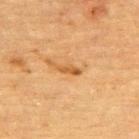Part of a total-body skin-imaging series; this lesion was reviewed on a skin check and was not flagged for biopsy. On the upper back. A female patient aged 68–72. The recorded lesion diameter is about 3 mm. This image is a 15 mm lesion crop taken from a total-body photograph. Automated tile analysis of the lesion measured a lesion area of about 3 mm², an eccentricity of roughly 0.9, and a shape-asymmetry score of about 0.35 (0 = symmetric). The software also gave a lesion color around L≈49 a*≈21 b*≈39 in CIELAB, about 9 CIELAB-L* units darker than the surrounding skin, and a normalized border contrast of about 7.5. It also reported a nevus-likeness score of about 25/100 and a detector confidence of about 90 out of 100 that the crop contains a lesion. Imaged with cross-polarized lighting.